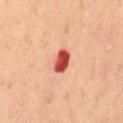<tbp_lesion>
<image>
  <source>total-body photography crop</source>
  <field_of_view_mm>15</field_of_view_mm>
</image>
<patient>
  <sex>female</sex>
  <age_approx>80</age_approx>
</patient>
<site>abdomen</site>
</tbp_lesion>